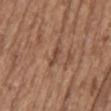Captured during whole-body skin photography for melanoma surveillance; the lesion was not biopsied. A 15 mm crop from a total-body photograph taken for skin-cancer surveillance. Longest diameter approximately 2.5 mm. Located on the mid back. Captured under white-light illumination. A female subject, about 65 years old.